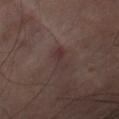Q: Was a biopsy performed?
A: total-body-photography surveillance lesion; no biopsy